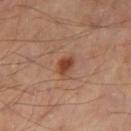This lesion was catalogued during total-body skin photography and was not selected for biopsy. About 2.5 mm across. From the right thigh. Captured under cross-polarized illumination. Cropped from a whole-body photographic skin survey; the tile spans about 15 mm.